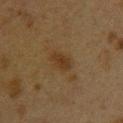Recorded during total-body skin imaging; not selected for excision or biopsy.
The lesion is on the left upper arm.
This is a cross-polarized tile.
The total-body-photography lesion software estimated an average lesion color of about L≈28 a*≈14 b*≈27 (CIELAB) and a normalized border contrast of about 7. The analysis additionally found an automated nevus-likeness rating near 60 out of 100 and lesion-presence confidence of about 100/100.
A female subject, roughly 40 years of age.
Cropped from a whole-body photographic skin survey; the tile spans about 15 mm.